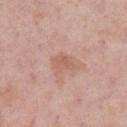The lesion was tiled from a total-body skin photograph and was not biopsied. A roughly 15 mm field-of-view crop from a total-body skin photograph. Automated tile analysis of the lesion measured a lesion color around L≈60 a*≈21 b*≈28 in CIELAB, a lesion–skin lightness drop of about 7, and a normalized lesion–skin contrast near 5.5. And it measured a border-irregularity rating of about 2.5/10, a within-lesion color-variation index near 2/10, and radial color variation of about 0.5. On the left lower leg. Captured under white-light illumination. A female subject, in their mid-50s.